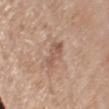image-analysis metrics=a lesion area of about 5.5 mm², an eccentricity of roughly 0.9, and two-axis asymmetry of about 0.3; a classifier nevus-likeness of about 0/100 and a detector confidence of about 100 out of 100 that the crop contains a lesion | patient=female, aged 83 or older | acquisition=15 mm crop, total-body photography | anatomic site=the left upper arm | tile lighting=white-light | lesion size=≈4 mm.